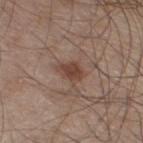No biopsy was performed on this lesion — it was imaged during a full skin examination and was not determined to be concerning. Imaged with white-light lighting. The patient is a male approximately 60 years of age. Automated image analysis of the tile measured a lesion area of about 5 mm², a shape eccentricity near 0.65, and a shape-asymmetry score of about 0.25 (0 = symmetric). The analysis additionally found a within-lesion color-variation index near 2.5/10 and radial color variation of about 1. And it measured a nevus-likeness score of about 90/100 and a lesion-detection confidence of about 100/100. A lesion tile, about 15 mm wide, cut from a 3D total-body photograph. The lesion is on the leg. About 3 mm across.